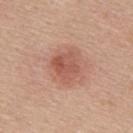biopsy status = imaged on a skin check; not biopsied | image = ~15 mm tile from a whole-body skin photo | tile lighting = white-light | size = ≈3.5 mm | site = the mid back | subject = male, roughly 55 years of age.